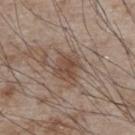biopsy status = no biopsy performed (imaged during a skin exam); subject = male, approximately 75 years of age; imaging modality = 15 mm crop, total-body photography; anatomic site = the chest.